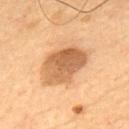– site — the upper back
– diameter — ≈6 mm
– image-analysis metrics — an area of roughly 18 mm², a shape eccentricity near 0.75, and a symmetry-axis asymmetry near 0.15; a mean CIELAB color near L≈56 a*≈20 b*≈37 and a lesion-to-skin contrast of about 8.5 (normalized; higher = more distinct); a nevus-likeness score of about 10/100 and a detector confidence of about 100 out of 100 that the crop contains a lesion
– tile lighting — cross-polarized illumination
– patient — male, aged 53–57
– acquisition — total-body-photography crop, ~15 mm field of view